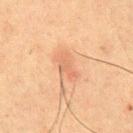The patient is a male about 50 years old. A 15 mm close-up tile from a total-body photography series done for melanoma screening. From the chest.